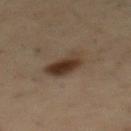Q: Was this lesion biopsied?
A: catalogued during a skin exam; not biopsied
Q: Lesion location?
A: the mid back
Q: Who is the patient?
A: male, in their mid-50s
Q: What is the imaging modality?
A: ~15 mm tile from a whole-body skin photo
Q: How was the tile lit?
A: cross-polarized